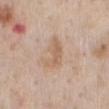Recorded during total-body skin imaging; not selected for excision or biopsy.
Captured under white-light illumination.
The subject is a male roughly 60 years of age.
The recorded lesion diameter is about 4.5 mm.
Automated tile analysis of the lesion measured an area of roughly 8.5 mm², an eccentricity of roughly 0.8, and two-axis asymmetry of about 0.4. The analysis additionally found an average lesion color of about L≈62 a*≈17 b*≈31 (CIELAB) and a lesion-to-skin contrast of about 6 (normalized; higher = more distinct). The software also gave a border-irregularity index near 4.5/10, internal color variation of about 2 on a 0–10 scale, and peripheral color asymmetry of about 0.5. And it measured a classifier nevus-likeness of about 0/100 and lesion-presence confidence of about 100/100.
A 15 mm close-up tile from a total-body photography series done for melanoma screening.
On the back.